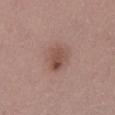The lesion was photographed on a routine skin check and not biopsied; there is no pathology result. On the right lower leg. About 3.5 mm across. Cropped from a total-body skin-imaging series; the visible field is about 15 mm. Captured under white-light illumination. An algorithmic analysis of the crop reported a lesion area of about 9 mm², a shape eccentricity near 0.55, and a shape-asymmetry score of about 0.15 (0 = symmetric). And it measured a mean CIELAB color near L≈51 a*≈20 b*≈24, a lesion–skin lightness drop of about 9, and a normalized lesion–skin contrast near 6.5. A female subject aged approximately 35.